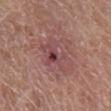The lesion was photographed on a routine skin check and not biopsied; there is no pathology result.